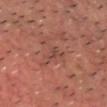The lesion was tiled from a total-body skin photograph and was not biopsied. This image is a 15 mm lesion crop taken from a total-body photograph. The recorded lesion diameter is about 2.5 mm. The subject is a male roughly 50 years of age. The tile uses cross-polarized illumination. On the head or neck.